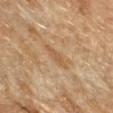Impression:
No biopsy was performed on this lesion — it was imaged during a full skin examination and was not determined to be concerning.
Image and clinical context:
The recorded lesion diameter is about 3.5 mm. A female patient aged approximately 70. From the right forearm. A 15 mm crop from a total-body photograph taken for skin-cancer surveillance.